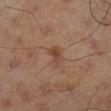Imaged during a routine full-body skin examination; the lesion was not biopsied and no histopathology is available.
Measured at roughly 3 mm in maximum diameter.
The lesion is located on the left lower leg.
A male patient, approximately 45 years of age.
Imaged with cross-polarized lighting.
A close-up tile cropped from a whole-body skin photograph, about 15 mm across.